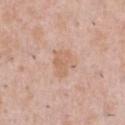No biopsy was performed on this lesion — it was imaged during a full skin examination and was not determined to be concerning.